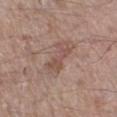The lesion was photographed on a routine skin check and not biopsied; there is no pathology result. A 15 mm close-up extracted from a 3D total-body photography capture. The patient is a male aged around 55. On the right lower leg. Captured under white-light illumination.